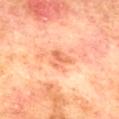follow-up: imaged on a skin check; not biopsied
image source: ~15 mm tile from a whole-body skin photo
subject: male, aged 73 to 77
location: the mid back